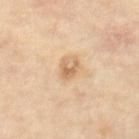<case>
  <biopsy_status>not biopsied; imaged during a skin examination</biopsy_status>
  <lesion_size>
    <long_diameter_mm_approx>3.0</long_diameter_mm_approx>
  </lesion_size>
  <automated_metrics>
    <cielab_L>66</cielab_L>
    <cielab_a>18</cielab_a>
    <cielab_b>37</cielab_b>
    <vs_skin_darker_L>10.0</vs_skin_darker_L>
    <vs_skin_contrast_norm>6.5</vs_skin_contrast_norm>
    <nevus_likeness_0_100>5</nevus_likeness_0_100>
  </automated_metrics>
  <patient>
    <sex>female</sex>
    <age_approx>75</age_approx>
  </patient>
  <image>
    <source>total-body photography crop</source>
    <field_of_view_mm>15</field_of_view_mm>
  </image>
  <site>leg</site>
  <lighting>cross-polarized</lighting>
</case>